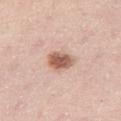follow-up = total-body-photography surveillance lesion; no biopsy.